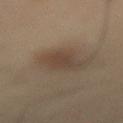follow-up = imaged on a skin check; not biopsied | patient = male, aged 53 to 57 | location = the lower back | tile lighting = cross-polarized | imaging modality = 15 mm crop, total-body photography | size = ≈3.5 mm.